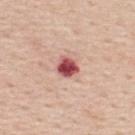Imaged during a routine full-body skin examination; the lesion was not biopsied and no histopathology is available. Approximately 2.5 mm at its widest. A 15 mm crop from a total-body photograph taken for skin-cancer surveillance. From the back. Imaged with white-light lighting. A male subject, aged approximately 60.